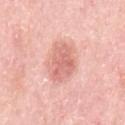Q: Is there a histopathology result?
A: imaged on a skin check; not biopsied
Q: Automated lesion metrics?
A: a lesion area of about 11 mm², a shape eccentricity near 0.75, and a shape-asymmetry score of about 0.25 (0 = symmetric); border irregularity of about 3 on a 0–10 scale, internal color variation of about 3 on a 0–10 scale, and radial color variation of about 1; a lesion-detection confidence of about 100/100
Q: Who is the patient?
A: female, approximately 65 years of age
Q: Lesion location?
A: the left upper arm
Q: What is the lesion's diameter?
A: ~4.5 mm (longest diameter)
Q: What kind of image is this?
A: ~15 mm tile from a whole-body skin photo